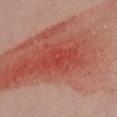Impression: The lesion was photographed on a routine skin check and not biopsied; there is no pathology result. Image and clinical context: Located on the right thigh. Automated image analysis of the tile measured a mean CIELAB color near L≈46 a*≈39 b*≈33, roughly 7 lightness units darker than nearby skin, and a normalized lesion–skin contrast near 6. It also reported a border-irregularity index near 4.5/10, a color-variation rating of about 2.5/10, and radial color variation of about 1. A close-up tile cropped from a whole-body skin photograph, about 15 mm across. Captured under white-light illumination. The lesion's longest dimension is about 5 mm. A female subject, approximately 30 years of age.